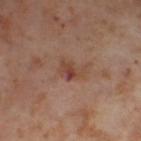Impression:
Captured during whole-body skin photography for melanoma surveillance; the lesion was not biopsied.
Acquisition and patient details:
A female patient, roughly 55 years of age. A lesion tile, about 15 mm wide, cut from a 3D total-body photograph. On the right thigh.